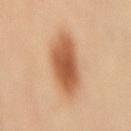{
  "biopsy_status": "not biopsied; imaged during a skin examination",
  "lighting": "cross-polarized",
  "image": {
    "source": "total-body photography crop",
    "field_of_view_mm": 15
  },
  "automated_metrics": {
    "eccentricity": 0.8,
    "shape_asymmetry": 0.15
  },
  "patient": {
    "sex": "female",
    "age_approx": 35
  },
  "lesion_size": {
    "long_diameter_mm_approx": 6.0
  },
  "site": "mid back"
}